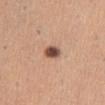| field | value |
|---|---|
| notes | imaged on a skin check; not biopsied |
| lighting | white-light |
| site | the abdomen |
| patient | female, aged 28 to 32 |
| acquisition | total-body-photography crop, ~15 mm field of view |
| lesion diameter | ~2.5 mm (longest diameter) |